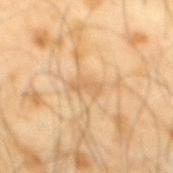image = ~15 mm tile from a whole-body skin photo | body site = the mid back | patient = male, about 65 years old | diameter = ≈3 mm.